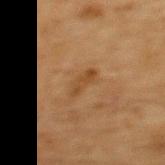Q: What is the anatomic site?
A: the upper back
Q: What are the patient's age and sex?
A: male, aged approximately 65
Q: What kind of image is this?
A: ~15 mm crop, total-body skin-cancer survey
Q: What lighting was used for the tile?
A: cross-polarized